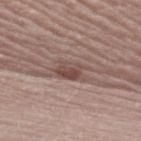Q: What did automated image analysis measure?
A: a nevus-likeness score of about 35/100 and lesion-presence confidence of about 90/100
Q: Patient demographics?
A: female, aged approximately 65
Q: What lighting was used for the tile?
A: white-light illumination
Q: What is the anatomic site?
A: the right upper arm
Q: How large is the lesion?
A: ~4.5 mm (longest diameter)
Q: What kind of image is this?
A: total-body-photography crop, ~15 mm field of view